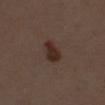<lesion>
<biopsy_status>not biopsied; imaged during a skin examination</biopsy_status>
<lighting>white-light</lighting>
<automated_metrics>
  <lesion_detection_confidence_0_100>100</lesion_detection_confidence_0_100>
</automated_metrics>
<image>
  <source>total-body photography crop</source>
  <field_of_view_mm>15</field_of_view_mm>
</image>
<lesion_size>
  <long_diameter_mm_approx>3.5</long_diameter_mm_approx>
</lesion_size>
<patient>
  <sex>female</sex>
  <age_approx>50</age_approx>
</patient>
<site>chest</site>
</lesion>